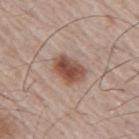Imaged during a routine full-body skin examination; the lesion was not biopsied and no histopathology is available. Automated tile analysis of the lesion measured a lesion area of about 8.5 mm², an outline eccentricity of about 0.75 (0 = round, 1 = elongated), and a shape-asymmetry score of about 0.15 (0 = symmetric). The analysis additionally found a mean CIELAB color near L≈49 a*≈20 b*≈27, a lesion–skin lightness drop of about 14, and a normalized border contrast of about 10. The analysis additionally found an automated nevus-likeness rating near 95 out of 100 and a detector confidence of about 100 out of 100 that the crop contains a lesion. A region of skin cropped from a whole-body photographic capture, roughly 15 mm wide. The subject is a male in their mid- to late 60s. The lesion is on the right upper arm. Imaged with white-light lighting.